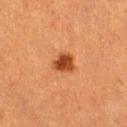biopsy_status: not biopsied; imaged during a skin examination
image:
  source: total-body photography crop
  field_of_view_mm: 15
lighting: cross-polarized
lesion_size:
  long_diameter_mm_approx: 2.5
patient:
  sex: female
  age_approx: 55
automated_metrics:
  cielab_L: 40
  cielab_a: 27
  cielab_b: 37
  vs_skin_darker_L: 14.0
  vs_skin_contrast_norm: 11.0
  border_irregularity_0_10: 2.5
  color_variation_0_10: 3.0
  nevus_likeness_0_100: 100
  lesion_detection_confidence_0_100: 100
site: left thigh